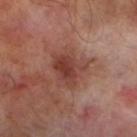{"biopsy_status": "not biopsied; imaged during a skin examination", "patient": {"sex": "male", "age_approx": 70}, "image": {"source": "total-body photography crop", "field_of_view_mm": 15}, "site": "left lower leg", "lighting": "cross-polarized", "lesion_size": {"long_diameter_mm_approx": 5.0}}